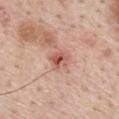No biopsy was performed on this lesion — it was imaged during a full skin examination and was not determined to be concerning. Measured at roughly 3 mm in maximum diameter. This is a white-light tile. A 15 mm close-up extracted from a 3D total-body photography capture. A male subject about 55 years old. Located on the mid back.